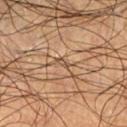Q: Was this lesion biopsied?
A: total-body-photography surveillance lesion; no biopsy
Q: How was the tile lit?
A: cross-polarized
Q: What is the anatomic site?
A: the front of the torso
Q: Automated lesion metrics?
A: a mean CIELAB color near L≈45 a*≈15 b*≈28, roughly 8 lightness units darker than nearby skin, and a normalized border contrast of about 6.5; a border-irregularity index near 3/10, internal color variation of about 0 on a 0–10 scale, and a peripheral color-asymmetry measure near 0; lesion-presence confidence of about 0/100
Q: What are the patient's age and sex?
A: male, aged 53 to 57
Q: What is the imaging modality?
A: ~15 mm crop, total-body skin-cancer survey